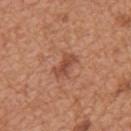body site: the mid back; lesion size: ≈3.5 mm; tile lighting: white-light; acquisition: total-body-photography crop, ~15 mm field of view; subject: male, aged approximately 65.